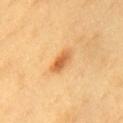Assessment:
This lesion was catalogued during total-body skin photography and was not selected for biopsy.
Context:
An algorithmic analysis of the crop reported an area of roughly 5 mm² and a shape eccentricity near 0.85. The software also gave a border-irregularity index near 2.5/10, a within-lesion color-variation index near 4/10, and radial color variation of about 1.5. And it measured an automated nevus-likeness rating near 95 out of 100. A male patient approximately 60 years of age. This image is a 15 mm lesion crop taken from a total-body photograph. Measured at roughly 3.5 mm in maximum diameter. Captured under cross-polarized illumination. On the chest.